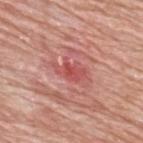The lesion was photographed on a routine skin check and not biopsied; there is no pathology result. A male patient aged 78–82. From the upper back. Cropped from a total-body skin-imaging series; the visible field is about 15 mm.